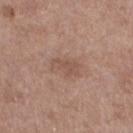Case summary:
* biopsy status · total-body-photography surveillance lesion; no biopsy
* body site · the left thigh
* image source · ~15 mm crop, total-body skin-cancer survey
* subject · female, aged approximately 65
* tile lighting · white-light illumination
* lesion diameter · ≈3 mm